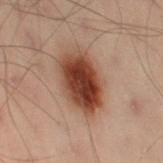biopsy_status: not biopsied; imaged during a skin examination
site: left leg
lesion_size:
  long_diameter_mm_approx: 6.0
image:
  source: total-body photography crop
  field_of_view_mm: 15
lighting: cross-polarized
patient:
  sex: male
  age_approx: 50